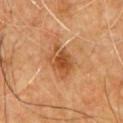Clinical impression:
The lesion was photographed on a routine skin check and not biopsied; there is no pathology result.
Background:
The lesion-visualizer software estimated an area of roughly 9 mm² and a shape-asymmetry score of about 0.3 (0 = symmetric). The lesion is on the chest. The tile uses cross-polarized illumination. A region of skin cropped from a whole-body photographic capture, roughly 15 mm wide. A male subject, approximately 60 years of age. The recorded lesion diameter is about 4 mm.